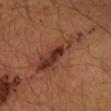follow-up — catalogued during a skin exam; not biopsied | lesion size — ~7.5 mm (longest diameter) | subject — male, aged 38 to 42 | TBP lesion metrics — an outline eccentricity of about 0.95 (0 = round, 1 = elongated) and a symmetry-axis asymmetry near 0.45; internal color variation of about 7 on a 0–10 scale; a nevus-likeness score of about 50/100 | acquisition — ~15 mm tile from a whole-body skin photo | location — the right forearm | tile lighting — cross-polarized.